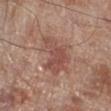| key | value |
|---|---|
| workup | imaged on a skin check; not biopsied |
| body site | the right lower leg |
| patient | male, aged 68–72 |
| image source | ~15 mm crop, total-body skin-cancer survey |
| lesion diameter | ≈6 mm |